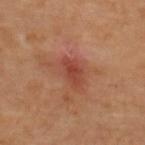Part of a total-body skin-imaging series; this lesion was reviewed on a skin check and was not flagged for biopsy. A female patient, approximately 70 years of age. Longest diameter approximately 3.5 mm. The tile uses cross-polarized illumination. A lesion tile, about 15 mm wide, cut from a 3D total-body photograph. Located on the back.